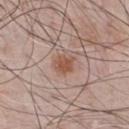Context: About 2.5 mm across. A 15 mm close-up extracted from a 3D total-body photography capture. On the chest. The subject is a male aged 63–67.